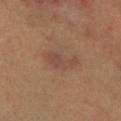body site: the right lower leg | acquisition: ~15 mm tile from a whole-body skin photo | patient: male, about 45 years old | size: ~4.5 mm (longest diameter).